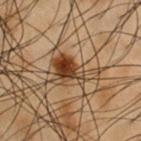<tbp_lesion>
<biopsy_status>not biopsied; imaged during a skin examination</biopsy_status>
<lighting>cross-polarized</lighting>
<patient>
  <sex>male</sex>
  <age_approx>55</age_approx>
</patient>
<lesion_size>
  <long_diameter_mm_approx>6.0</long_diameter_mm_approx>
</lesion_size>
<image>
  <source>total-body photography crop</source>
  <field_of_view_mm>15</field_of_view_mm>
</image>
<automated_metrics>
  <area_mm2_approx>13.0</area_mm2_approx>
  <eccentricity>0.8</eccentricity>
  <shape_asymmetry>0.45</shape_asymmetry>
  <nevus_likeness_0_100>100</nevus_likeness_0_100>
  <lesion_detection_confidence_0_100>100</lesion_detection_confidence_0_100>
</automated_metrics>
<site>chest</site>
</tbp_lesion>